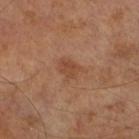Recorded during total-body skin imaging; not selected for excision or biopsy.
A male subject, aged around 70.
This is a cross-polarized tile.
This image is a 15 mm lesion crop taken from a total-body photograph.
The lesion's longest dimension is about 3 mm.
The total-body-photography lesion software estimated an area of roughly 5 mm², an eccentricity of roughly 0.6, and a symmetry-axis asymmetry near 0.3. The software also gave a classifier nevus-likeness of about 15/100 and lesion-presence confidence of about 100/100.
Located on the right lower leg.